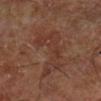Q: Was this lesion biopsied?
A: catalogued during a skin exam; not biopsied
Q: Lesion location?
A: the right lower leg
Q: Who is the patient?
A: approximately 65 years of age
Q: Lesion size?
A: ≈8 mm
Q: How was this image acquired?
A: ~15 mm tile from a whole-body skin photo